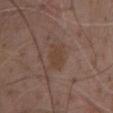Recorded during total-body skin imaging; not selected for excision or biopsy. The total-body-photography lesion software estimated a lesion color around L≈41 a*≈17 b*≈26 in CIELAB, roughly 5 lightness units darker than nearby skin, and a normalized lesion–skin contrast near 6. And it measured a border-irregularity rating of about 2.5/10, internal color variation of about 1.5 on a 0–10 scale, and a peripheral color-asymmetry measure near 0.5. The software also gave a classifier nevus-likeness of about 0/100 and lesion-presence confidence of about 100/100. A male subject aged 68–72. The recorded lesion diameter is about 3 mm. A close-up tile cropped from a whole-body skin photograph, about 15 mm across. Captured under white-light illumination. Located on the chest.